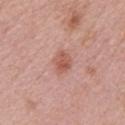Imaged during a routine full-body skin examination; the lesion was not biopsied and no histopathology is available. Captured under white-light illumination. The lesion is on the right upper arm. This image is a 15 mm lesion crop taken from a total-body photograph. A female patient, approximately 65 years of age. Approximately 2.5 mm at its widest.